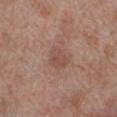workup=catalogued during a skin exam; not biopsied
patient=male, aged approximately 70
site=the left lower leg
tile lighting=white-light
TBP lesion metrics=a symmetry-axis asymmetry near 0.25; a classifier nevus-likeness of about 0/100 and a lesion-detection confidence of about 100/100
image=15 mm crop, total-body photography
lesion diameter=≈3 mm